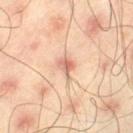Assessment: This lesion was catalogued during total-body skin photography and was not selected for biopsy. Image and clinical context: The patient is a male approximately 45 years of age. A close-up tile cropped from a whole-body skin photograph, about 15 mm across. The lesion is on the left thigh. The recorded lesion diameter is about 3 mm. This is a cross-polarized tile.